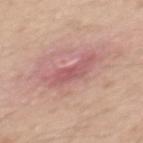Assessment:
Recorded during total-body skin imaging; not selected for excision or biopsy.
Clinical summary:
Located on the mid back. A male subject aged 43–47. A 15 mm close-up extracted from a 3D total-body photography capture.